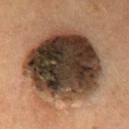This lesion was catalogued during total-body skin photography and was not selected for biopsy. The tile uses cross-polarized illumination. A male patient, about 55 years old. On the chest. Automated tile analysis of the lesion measured an average lesion color of about L≈33 a*≈13 b*≈23 (CIELAB) and a lesion-to-skin contrast of about 17.5 (normalized; higher = more distinct). A 15 mm close-up tile from a total-body photography series done for melanoma screening. Longest diameter approximately 10 mm.